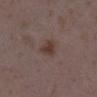Assessment: Part of a total-body skin-imaging series; this lesion was reviewed on a skin check and was not flagged for biopsy. Clinical summary: A 15 mm close-up extracted from a 3D total-body photography capture. The lesion's longest dimension is about 2.5 mm. The patient is a female about 35 years old. The lesion is located on the right lower leg. Captured under white-light illumination.